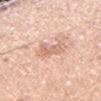notes: total-body-photography surveillance lesion; no biopsy
patient: male, aged around 45
site: the right upper arm
tile lighting: white-light
image source: total-body-photography crop, ~15 mm field of view
TBP lesion metrics: an area of roughly 4.5 mm², an outline eccentricity of about 0.6 (0 = round, 1 = elongated), and two-axis asymmetry of about 0.35; a lesion–skin lightness drop of about 9 and a normalized border contrast of about 5.5; a peripheral color-asymmetry measure near 1